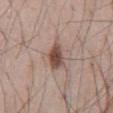• biopsy status: no biopsy performed (imaged during a skin exam)